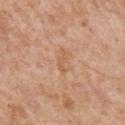The lesion was tiled from a total-body skin photograph and was not biopsied. A male subject roughly 65 years of age. The tile uses white-light illumination. A roughly 15 mm field-of-view crop from a total-body skin photograph. The lesion is located on the chest. The lesion's longest dimension is about 2.5 mm.